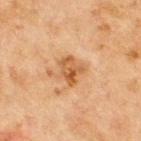<case>
  <biopsy_status>not biopsied; imaged during a skin examination</biopsy_status>
  <patient>
    <sex>male</sex>
    <age_approx>65</age_approx>
  </patient>
  <lesion_size>
    <long_diameter_mm_approx>3.0</long_diameter_mm_approx>
  </lesion_size>
  <image>
    <source>total-body photography crop</source>
    <field_of_view_mm>15</field_of_view_mm>
  </image>
  <lighting>cross-polarized</lighting>
  <site>front of the torso</site>
</case>